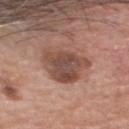notes = total-body-photography surveillance lesion; no biopsy
patient = male, aged around 60
site = the head or neck
image source = ~15 mm tile from a whole-body skin photo